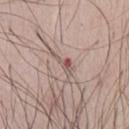The tile uses white-light illumination.
The lesion is on the chest.
A male patient, aged approximately 65.
A region of skin cropped from a whole-body photographic capture, roughly 15 mm wide.
Automated image analysis of the tile measured about 9 CIELAB-L* units darker than the surrounding skin. It also reported a border-irregularity index near 6.5/10, a color-variation rating of about 5/10, and peripheral color asymmetry of about 1.